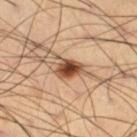This lesion was catalogued during total-body skin photography and was not selected for biopsy. A male subject about 65 years old. The recorded lesion diameter is about 4 mm. This is a cross-polarized tile. A 15 mm close-up tile from a total-body photography series done for melanoma screening. Automated image analysis of the tile measured a border-irregularity index near 3.5/10, internal color variation of about 6 on a 0–10 scale, and peripheral color asymmetry of about 1.5. On the right thigh.